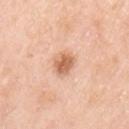Context: Captured under white-light illumination. Approximately 3 mm at its widest. A close-up tile cropped from a whole-body skin photograph, about 15 mm across. A female subject, roughly 50 years of age. The lesion is located on the left upper arm.